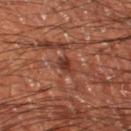Acquisition and patient details:
A 15 mm close-up extracted from a 3D total-body photography capture. The patient is a male aged 58 to 62. Approximately 2 mm at its widest. On the leg. This is a cross-polarized tile.